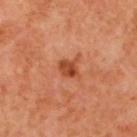Impression:
Part of a total-body skin-imaging series; this lesion was reviewed on a skin check and was not flagged for biopsy.
Background:
From the upper back. Captured under cross-polarized illumination. The lesion-visualizer software estimated a lesion area of about 4 mm², an outline eccentricity of about 0.55 (0 = round, 1 = elongated), and a symmetry-axis asymmetry near 0.2. The analysis additionally found a border-irregularity rating of about 2.5/10, internal color variation of about 4.5 on a 0–10 scale, and peripheral color asymmetry of about 1.5. A 15 mm close-up tile from a total-body photography series done for melanoma screening. Approximately 2 mm at its widest. A male patient aged approximately 70.